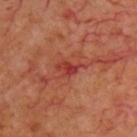<tbp_lesion>
  <biopsy_status>not biopsied; imaged during a skin examination</biopsy_status>
  <lesion_size>
    <long_diameter_mm_approx>3.5</long_diameter_mm_approx>
  </lesion_size>
  <site>chest</site>
  <patient>
    <sex>male</sex>
    <age_approx>70</age_approx>
  </patient>
  <image>
    <source>total-body photography crop</source>
    <field_of_view_mm>15</field_of_view_mm>
  </image>
</tbp_lesion>